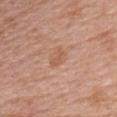Recorded during total-body skin imaging; not selected for excision or biopsy.
On the upper back.
The patient is a female in their 50s.
Automated tile analysis of the lesion measured an area of roughly 2.5 mm², an outline eccentricity of about 0.9 (0 = round, 1 = elongated), and two-axis asymmetry of about 0.5. It also reported an average lesion color of about L≈57 a*≈22 b*≈33 (CIELAB), roughly 7 lightness units darker than nearby skin, and a normalized lesion–skin contrast near 5.5. The analysis additionally found border irregularity of about 5 on a 0–10 scale, a color-variation rating of about 0/10, and peripheral color asymmetry of about 0. The analysis additionally found a nevus-likeness score of about 0/100.
Imaged with white-light lighting.
Cropped from a whole-body photographic skin survey; the tile spans about 15 mm.